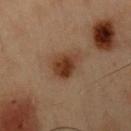{"site": "chest", "patient": {"sex": "male", "age_approx": 55}, "image": {"source": "total-body photography crop", "field_of_view_mm": 15}, "lighting": "cross-polarized", "lesion_size": {"long_diameter_mm_approx": 3.5}}